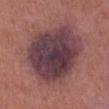<lesion>
<biopsy_status>not biopsied; imaged during a skin examination</biopsy_status>
<automated_metrics>
  <cielab_L>39</cielab_L>
  <cielab_a>21</cielab_a>
  <cielab_b>13</cielab_b>
  <vs_skin_darker_L>14.0</vs_skin_darker_L>
  <border_irregularity_0_10>2.0</border_irregularity_0_10>
  <peripheral_color_asymmetry>2.0</peripheral_color_asymmetry>
  <nevus_likeness_0_100>0</nevus_likeness_0_100>
  <lesion_detection_confidence_0_100>95</lesion_detection_confidence_0_100>
</automated_metrics>
<lesion_size>
  <long_diameter_mm_approx>9.5</long_diameter_mm_approx>
</lesion_size>
<image>
  <source>total-body photography crop</source>
  <field_of_view_mm>15</field_of_view_mm>
</image>
<patient>
  <sex>male</sex>
  <age_approx>85+</age_approx>
</patient>
<site>left lower leg</site>
<lighting>white-light</lighting>
</lesion>